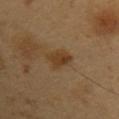workup = imaged on a skin check; not biopsied.